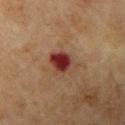Q: Was this lesion biopsied?
A: no biopsy performed (imaged during a skin exam)
Q: What are the patient's age and sex?
A: female, aged 68–72
Q: How large is the lesion?
A: ≈3.5 mm
Q: How was the tile lit?
A: cross-polarized illumination
Q: Lesion location?
A: the left upper arm
Q: What is the imaging modality?
A: total-body-photography crop, ~15 mm field of view